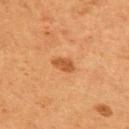| key | value |
|---|---|
| biopsy status | total-body-photography surveillance lesion; no biopsy |
| imaging modality | ~15 mm tile from a whole-body skin photo |
| size | about 3 mm |
| tile lighting | cross-polarized |
| subject | male, in their mid- to late 50s |
| location | the upper back |